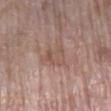{"biopsy_status": "not biopsied; imaged during a skin examination", "patient": {"sex": "female", "age_approx": 75}, "site": "leg", "lighting": "white-light", "image": {"source": "total-body photography crop", "field_of_view_mm": 15}, "lesion_size": {"long_diameter_mm_approx": 4.0}}